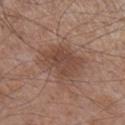The lesion's longest dimension is about 5 mm. This is a white-light tile. The patient is a male about 55 years old. From the right lower leg. A region of skin cropped from a whole-body photographic capture, roughly 15 mm wide.